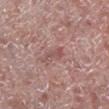biopsy status=total-body-photography surveillance lesion; no biopsy | lighting=white-light | size=≈3 mm | automated lesion analysis=a classifier nevus-likeness of about 0/100 and a detector confidence of about 95 out of 100 that the crop contains a lesion | image=~15 mm tile from a whole-body skin photo | location=the right lower leg | patient=male, in their mid-60s.